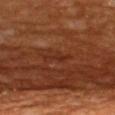Recorded during total-body skin imaging; not selected for excision or biopsy.
The patient is a male aged 58 to 62.
From the upper back.
Longest diameter approximately 3.5 mm.
Captured under cross-polarized illumination.
A region of skin cropped from a whole-body photographic capture, roughly 15 mm wide.
The lesion-visualizer software estimated a footprint of about 3.5 mm², a shape eccentricity near 0.95, and two-axis asymmetry of about 0.45. The analysis additionally found a mean CIELAB color near L≈27 a*≈24 b*≈29, about 5 CIELAB-L* units darker than the surrounding skin, and a lesion-to-skin contrast of about 6 (normalized; higher = more distinct). It also reported a border-irregularity rating of about 6/10, a color-variation rating of about 0/10, and peripheral color asymmetry of about 0. It also reported an automated nevus-likeness rating near 0 out of 100.